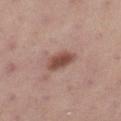biopsy_status: not biopsied; imaged during a skin examination
image:
  source: total-body photography crop
  field_of_view_mm: 15
patient:
  sex: female
  age_approx: 25
site: left lower leg
lesion_size:
  long_diameter_mm_approx: 3.5